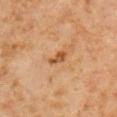Clinical impression:
Captured during whole-body skin photography for melanoma surveillance; the lesion was not biopsied.
Background:
Longest diameter approximately 3 mm. This is a cross-polarized tile. Cropped from a whole-body photographic skin survey; the tile spans about 15 mm. On the arm. The total-body-photography lesion software estimated a border-irregularity index near 3/10 and a color-variation rating of about 1.5/10. The patient is a male roughly 60 years of age.